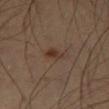<case>
  <biopsy_status>not biopsied; imaged during a skin examination</biopsy_status>
  <lighting>cross-polarized</lighting>
  <image>
    <source>total-body photography crop</source>
    <field_of_view_mm>15</field_of_view_mm>
  </image>
  <patient>
    <sex>male</sex>
    <age_approx>55</age_approx>
  </patient>
  <site>left thigh</site>
  <automated_metrics>
    <eccentricity>0.9</eccentricity>
    <shape_asymmetry>0.4</shape_asymmetry>
    <cielab_L>33</cielab_L>
    <cielab_a>17</cielab_a>
    <cielab_b>25</cielab_b>
    <vs_skin_darker_L>8.0</vs_skin_darker_L>
    <vs_skin_contrast_norm>8.0</vs_skin_contrast_norm>
    <border_irregularity_0_10>3.5</border_irregularity_0_10>
    <color_variation_0_10>1.0</color_variation_0_10>
    <peripheral_color_asymmetry>0.0</peripheral_color_asymmetry>
    <nevus_likeness_0_100>95</nevus_likeness_0_100>
    <lesion_detection_confidence_0_100>100</lesion_detection_confidence_0_100>
  </automated_metrics>
  <lesion_size>
    <long_diameter_mm_approx>2.5</long_diameter_mm_approx>
  </lesion_size>
</case>